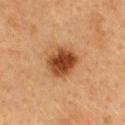Q: Was this lesion biopsied?
A: no biopsy performed (imaged during a skin exam)
Q: Lesion size?
A: about 4 mm
Q: Lesion location?
A: the back
Q: Who is the patient?
A: female, roughly 60 years of age
Q: How was this image acquired?
A: total-body-photography crop, ~15 mm field of view
Q: What lighting was used for the tile?
A: cross-polarized